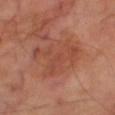Findings:
• workup — no biopsy performed (imaged during a skin exam)
• acquisition — ~15 mm crop, total-body skin-cancer survey
• anatomic site — the right thigh
• subject — male, in their 70s